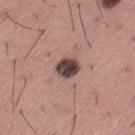<case>
<biopsy_status>not biopsied; imaged during a skin examination</biopsy_status>
<site>left thigh</site>
<lighting>white-light</lighting>
<image>
  <source>total-body photography crop</source>
  <field_of_view_mm>15</field_of_view_mm>
</image>
<lesion_size>
  <long_diameter_mm_approx>2.5</long_diameter_mm_approx>
</lesion_size>
<patient>
  <sex>male</sex>
  <age_approx>45</age_approx>
</patient>
</case>